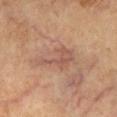Findings:
* biopsy status · no biopsy performed (imaged during a skin exam)
* acquisition · total-body-photography crop, ~15 mm field of view
* image-analysis metrics · a lesion color around L≈50 a*≈20 b*≈26 in CIELAB, about 7 CIELAB-L* units darker than the surrounding skin, and a normalized border contrast of about 5.5; a border-irregularity rating of about 6/10, a within-lesion color-variation index near 2.5/10, and radial color variation of about 1
* tile lighting · cross-polarized
* site · the chest
* lesion diameter · ~5 mm (longest diameter)
* patient · female, aged approximately 60